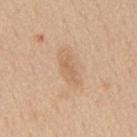Case summary:
– biopsy status: total-body-photography surveillance lesion; no biopsy
– subject: male, aged 58 to 62
– anatomic site: the mid back
– acquisition: ~15 mm crop, total-body skin-cancer survey